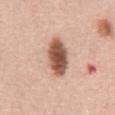Clinical summary: Measured at roughly 5 mm in maximum diameter. The tile uses white-light illumination. On the abdomen. A 15 mm close-up tile from a total-body photography series done for melanoma screening. The subject is a male aged 48 to 52.